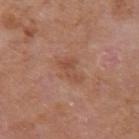Assessment: Imaged during a routine full-body skin examination; the lesion was not biopsied and no histopathology is available. Background: Measured at roughly 3.5 mm in maximum diameter. The lesion is located on the left upper arm. Imaged with white-light lighting. The patient is a male approximately 65 years of age. Cropped from a total-body skin-imaging series; the visible field is about 15 mm.